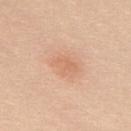– biopsy status · no biopsy performed (imaged during a skin exam)
– lighting · white-light illumination
– image · 15 mm crop, total-body photography
– lesion size · ≈3 mm
– image-analysis metrics · a border-irregularity index near 3/10, a color-variation rating of about 2/10, and a peripheral color-asymmetry measure near 0.5; a nevus-likeness score of about 25/100 and lesion-presence confidence of about 100/100
– site · the upper back
– subject · female, aged 18–22